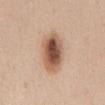follow-up — total-body-photography surveillance lesion; no biopsy
image source — ~15 mm tile from a whole-body skin photo
size — about 5.5 mm
site — the abdomen
tile lighting — white-light illumination
subject — female, approximately 30 years of age
automated metrics — a lesion area of about 14 mm², an eccentricity of roughly 0.8, and a symmetry-axis asymmetry near 0.15; a lesion color around L≈55 a*≈20 b*≈30 in CIELAB, a lesion–skin lightness drop of about 17, and a normalized lesion–skin contrast near 11; a border-irregularity index near 1.5/10, internal color variation of about 9.5 on a 0–10 scale, and radial color variation of about 3; a classifier nevus-likeness of about 100/100